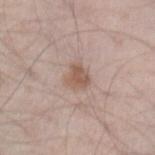This lesion was catalogued during total-body skin photography and was not selected for biopsy. The patient is a male approximately 45 years of age. Longest diameter approximately 2.5 mm. A region of skin cropped from a whole-body photographic capture, roughly 15 mm wide. Imaged with white-light lighting. From the left forearm. Automated tile analysis of the lesion measured about 9 CIELAB-L* units darker than the surrounding skin. The analysis additionally found border irregularity of about 2.5 on a 0–10 scale, a within-lesion color-variation index near 2.5/10, and radial color variation of about 1. The software also gave an automated nevus-likeness rating near 50 out of 100 and a lesion-detection confidence of about 100/100.